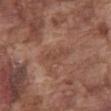Recorded during total-body skin imaging; not selected for excision or biopsy.
The total-body-photography lesion software estimated a footprint of about 3.5 mm² and a shape-asymmetry score of about 0.45 (0 = symmetric). The analysis additionally found a color-variation rating of about 0/10 and peripheral color asymmetry of about 0. And it measured a nevus-likeness score of about 0/100 and lesion-presence confidence of about 95/100.
The patient is a male about 75 years old.
Cropped from a whole-body photographic skin survey; the tile spans about 15 mm.
The tile uses white-light illumination.
The lesion's longest dimension is about 3.5 mm.
From the abdomen.